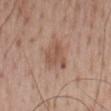The lesion was photographed on a routine skin check and not biopsied; there is no pathology result. Cropped from a whole-body photographic skin survey; the tile spans about 15 mm. This is a white-light tile. Located on the back. A male patient, aged 68–72. The lesion-visualizer software estimated a lesion area of about 8.5 mm², an outline eccentricity of about 0.7 (0 = round, 1 = elongated), and a symmetry-axis asymmetry near 0.35. And it measured a lesion color around L≈53 a*≈20 b*≈28 in CIELAB, roughly 8 lightness units darker than nearby skin, and a normalized border contrast of about 6. And it measured a nevus-likeness score of about 25/100 and a lesion-detection confidence of about 100/100.